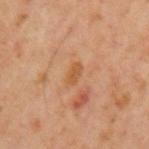Imaged during a routine full-body skin examination; the lesion was not biopsied and no histopathology is available.
Measured at roughly 2.5 mm in maximum diameter.
A male patient, aged 58 to 62.
Located on the left upper arm.
Cropped from a total-body skin-imaging series; the visible field is about 15 mm.
This is a cross-polarized tile.